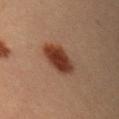A 15 mm close-up tile from a total-body photography series done for melanoma screening. Imaged with cross-polarized lighting. From the right upper arm. The subject is a male roughly 40 years of age.